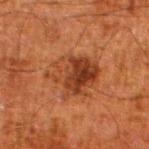Assessment: Imaged during a routine full-body skin examination; the lesion was not biopsied and no histopathology is available.